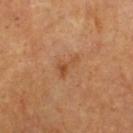The lesion is on the front of the torso. A lesion tile, about 15 mm wide, cut from a 3D total-body photograph. A male patient aged approximately 60.